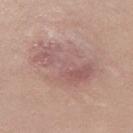No biopsy was performed on this lesion — it was imaged during a full skin examination and was not determined to be concerning.
A female subject, aged approximately 20.
Cropped from a total-body skin-imaging series; the visible field is about 15 mm.
Imaged with white-light lighting.
Approximately 7 mm at its widest.
Located on the left thigh.